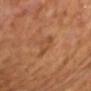A male subject approximately 60 years of age. A 15 mm crop from a total-body photograph taken for skin-cancer surveillance. Approximately 4.5 mm at its widest. An algorithmic analysis of the crop reported an outline eccentricity of about 0.9 (0 = round, 1 = elongated) and a shape-asymmetry score of about 0.4 (0 = symmetric). The analysis additionally found roughly 6 lightness units darker than nearby skin and a normalized lesion–skin contrast near 5. And it measured border irregularity of about 5.5 on a 0–10 scale, a color-variation rating of about 2.5/10, and a peripheral color-asymmetry measure near 1. On the upper back. The tile uses cross-polarized illumination.